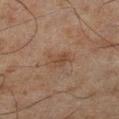Assessment:
The lesion was tiled from a total-body skin photograph and was not biopsied.
Acquisition and patient details:
From the left lower leg. Longest diameter approximately 2.5 mm. A 15 mm crop from a total-body photograph taken for skin-cancer surveillance. The patient is a male aged 43 to 47.